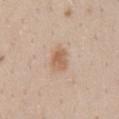biopsy status = total-body-photography surveillance lesion; no biopsy | size = ≈3 mm | subject = female, in their mid- to late 40s | illumination = white-light illumination | image = total-body-photography crop, ~15 mm field of view | location = the mid back.